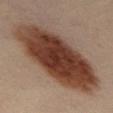This is a cross-polarized tile. Approximately 12 mm at its widest. Automated image analysis of the tile measured a lesion color around L≈39 a*≈20 b*≈26 in CIELAB, a lesion–skin lightness drop of about 17, and a lesion-to-skin contrast of about 13.5 (normalized; higher = more distinct). And it measured a classifier nevus-likeness of about 100/100. The patient is a male roughly 50 years of age. Located on the abdomen. A 15 mm crop from a total-body photograph taken for skin-cancer surveillance.